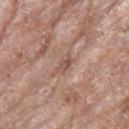biopsy_status: not biopsied; imaged during a skin examination
patient:
  sex: female
  age_approx: 75
image:
  source: total-body photography crop
  field_of_view_mm: 15
automated_metrics:
  area_mm2_approx: 3.0
  eccentricity: 0.85
  cielab_L: 52
  cielab_a: 20
  cielab_b: 25
  vs_skin_darker_L: 9.0
  vs_skin_contrast_norm: 6.0
  lesion_detection_confidence_0_100: 70
site: right thigh
lighting: white-light